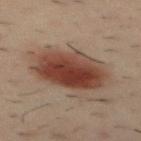A 15 mm crop from a total-body photograph taken for skin-cancer surveillance. From the mid back. Automated image analysis of the tile measured an area of roughly 30 mm², an eccentricity of roughly 0.75, and a shape-asymmetry score of about 0.15 (0 = symmetric). And it measured about 13 CIELAB-L* units darker than the surrounding skin. A male subject roughly 40 years of age. This is a cross-polarized tile. Longest diameter approximately 7.5 mm.